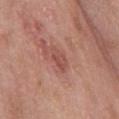Context: The lesion's longest dimension is about 2.5 mm. A region of skin cropped from a whole-body photographic capture, roughly 15 mm wide. A male subject in their mid- to late 50s. On the chest. Captured under white-light illumination.